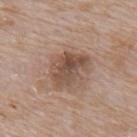Assessment: Part of a total-body skin-imaging series; this lesion was reviewed on a skin check and was not flagged for biopsy. Image and clinical context: This image is a 15 mm lesion crop taken from a total-body photograph. Measured at roughly 5 mm in maximum diameter. From the upper back. An algorithmic analysis of the crop reported an automated nevus-likeness rating near 15 out of 100 and lesion-presence confidence of about 100/100. Captured under white-light illumination. A male patient aged approximately 65.